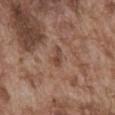The lesion was tiled from a total-body skin photograph and was not biopsied.
The lesion is located on the front of the torso.
A 15 mm crop from a total-body photograph taken for skin-cancer surveillance.
An algorithmic analysis of the crop reported a lesion area of about 2.5 mm², an eccentricity of roughly 0.9, and a symmetry-axis asymmetry near 0.45. The analysis additionally found a border-irregularity index near 4.5/10. And it measured a classifier nevus-likeness of about 0/100 and a detector confidence of about 95 out of 100 that the crop contains a lesion.
About 3 mm across.
A male patient, aged 73 to 77.
This is a white-light tile.